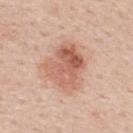Case summary:
– notes · no biopsy performed (imaged during a skin exam)
– anatomic site · the mid back
– lighting · white-light
– subject · male, aged 58 to 62
– image source · total-body-photography crop, ~15 mm field of view